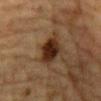The lesion was photographed on a routine skin check and not biopsied; there is no pathology result. Imaged with cross-polarized lighting. Automated image analysis of the tile measured a footprint of about 8 mm², an eccentricity of roughly 0.8, and two-axis asymmetry of about 0.2. The software also gave a border-irregularity rating of about 2/10, internal color variation of about 4.5 on a 0–10 scale, and a peripheral color-asymmetry measure near 1.5. The software also gave lesion-presence confidence of about 100/100. A male patient, about 85 years old or older. From the chest. Approximately 4 mm at its widest. A close-up tile cropped from a whole-body skin photograph, about 15 mm across.